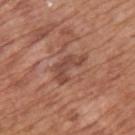The lesion was photographed on a routine skin check and not biopsied; there is no pathology result.
The total-body-photography lesion software estimated a peripheral color-asymmetry measure near 1. It also reported a classifier nevus-likeness of about 0/100.
The lesion is located on the upper back.
The patient is a male aged around 65.
A roughly 15 mm field-of-view crop from a total-body skin photograph.
Longest diameter approximately 4.5 mm.
The tile uses white-light illumination.